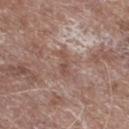Q: Was a biopsy performed?
A: no biopsy performed (imaged during a skin exam)
Q: How was the tile lit?
A: white-light
Q: What kind of image is this?
A: 15 mm crop, total-body photography
Q: What are the patient's age and sex?
A: male, in their 60s
Q: What is the anatomic site?
A: the right lower leg
Q: What is the lesion's diameter?
A: about 3.5 mm
Q: Automated lesion metrics?
A: a border-irregularity rating of about 5.5/10, a color-variation rating of about 0/10, and peripheral color asymmetry of about 0; an automated nevus-likeness rating near 0 out of 100